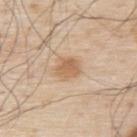Captured during whole-body skin photography for melanoma surveillance; the lesion was not biopsied. A male subject, about 80 years old. A close-up tile cropped from a whole-body skin photograph, about 15 mm across. Imaged with white-light lighting. The lesion is located on the upper back. An algorithmic analysis of the crop reported an average lesion color of about L≈62 a*≈18 b*≈35 (CIELAB) and a lesion–skin lightness drop of about 10. It also reported border irregularity of about 2 on a 0–10 scale, a color-variation rating of about 2.5/10, and peripheral color asymmetry of about 1. The analysis additionally found a nevus-likeness score of about 90/100 and a lesion-detection confidence of about 100/100.